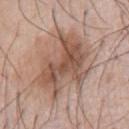follow-up: imaged on a skin check; not biopsied
lesion diameter: ≈8 mm
tile lighting: white-light
body site: the front of the torso
acquisition: 15 mm crop, total-body photography
subject: male, aged 73 to 77